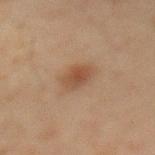Case summary:
• follow-up: imaged on a skin check; not biopsied
• image: ~15 mm crop, total-body skin-cancer survey
• lighting: cross-polarized
• anatomic site: the front of the torso
• automated metrics: a border-irregularity index near 2/10 and peripheral color asymmetry of about 1
• subject: male, aged 58 to 62
• lesion size: ~3 mm (longest diameter)